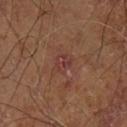{"biopsy_status": "not biopsied; imaged during a skin examination", "automated_metrics": {"area_mm2_approx": 4.0, "shape_asymmetry": 0.25, "cielab_L": 35, "cielab_a": 22, "cielab_b": 22, "vs_skin_darker_L": 5.0, "vs_skin_contrast_norm": 6.0, "nevus_likeness_0_100": 0}, "patient": {"sex": "male", "age_approx": 60}, "lesion_size": {"long_diameter_mm_approx": 2.5}, "image": {"source": "total-body photography crop", "field_of_view_mm": 15}, "site": "leg"}